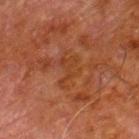Impression: No biopsy was performed on this lesion — it was imaged during a full skin examination and was not determined to be concerning. Clinical summary: Longest diameter approximately 4 mm. The total-body-photography lesion software estimated a footprint of about 6 mm², a shape eccentricity near 0.85, and a shape-asymmetry score of about 0.55 (0 = symmetric). From the right lower leg. A male patient aged 78–82. Cropped from a whole-body photographic skin survey; the tile spans about 15 mm.